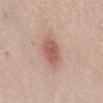biopsy_status: not biopsied; imaged during a skin examination
image:
  source: total-body photography crop
  field_of_view_mm: 15
site: lower back
patient:
  sex: female
  age_approx: 20